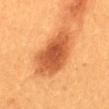Recorded during total-body skin imaging; not selected for excision or biopsy.
A female patient aged around 30.
This image is a 15 mm lesion crop taken from a total-body photograph.
Automated image analysis of the tile measured an area of roughly 23 mm².
Approximately 7.5 mm at its widest.
Captured under cross-polarized illumination.
Located on the back.